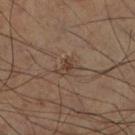This lesion was catalogued during total-body skin photography and was not selected for biopsy.
About 2.5 mm across.
The subject is a male about 60 years old.
This is a cross-polarized tile.
On the right lower leg.
A roughly 15 mm field-of-view crop from a total-body skin photograph.